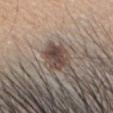Assessment: Recorded during total-body skin imaging; not selected for excision or biopsy. Background: Cropped from a whole-body photographic skin survey; the tile spans about 15 mm. A male subject roughly 40 years of age. Located on the head or neck.